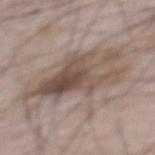The lesion was tiled from a total-body skin photograph and was not biopsied.
This is a white-light tile.
A male patient in their mid-60s.
The lesion's longest dimension is about 10 mm.
From the mid back.
A 15 mm close-up extracted from a 3D total-body photography capture.